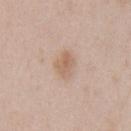biopsy_status: not biopsied; imaged during a skin examination
site: chest
lesion_size:
  long_diameter_mm_approx: 3.0
image:
  source: total-body photography crop
  field_of_view_mm: 15
automated_metrics:
  area_mm2_approx: 5.5
  eccentricity: 0.75
  shape_asymmetry: 0.25
  cielab_L: 62
  cielab_a: 17
  cielab_b: 30
  vs_skin_darker_L: 8.0
  vs_skin_contrast_norm: 6.0
lighting: white-light
patient:
  sex: male
  age_approx: 50